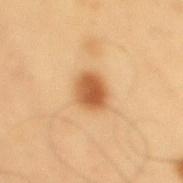Findings:
* notes · catalogued during a skin exam; not biopsied
* location · the mid back
* subject · male, approximately 55 years of age
* lesion diameter · about 3.5 mm
* acquisition · 15 mm crop, total-body photography